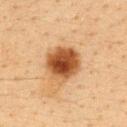biopsy status=imaged on a skin check; not biopsied
body site=the upper back
subject=female, aged around 40
acquisition=total-body-photography crop, ~15 mm field of view